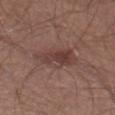Captured during whole-body skin photography for melanoma surveillance; the lesion was not biopsied. Captured under white-light illumination. Measured at roughly 5.5 mm in maximum diameter. A male patient, aged approximately 25. Cropped from a total-body skin-imaging series; the visible field is about 15 mm. The lesion is on the left thigh.